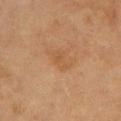Q: Lesion size?
A: about 3 mm
Q: Who is the patient?
A: female, about 80 years old
Q: What is the anatomic site?
A: the arm
Q: What is the imaging modality?
A: ~15 mm crop, total-body skin-cancer survey